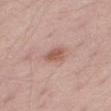The lesion was tiled from a total-body skin photograph and was not biopsied.
Automated tile analysis of the lesion measured a footprint of about 4 mm², an outline eccentricity of about 0.75 (0 = round, 1 = elongated), and two-axis asymmetry of about 0.3. And it measured a mean CIELAB color near L≈56 a*≈23 b*≈26, roughly 11 lightness units darker than nearby skin, and a lesion-to-skin contrast of about 7.5 (normalized; higher = more distinct). It also reported a border-irregularity rating of about 2.5/10 and a peripheral color-asymmetry measure near 0.5. The software also gave a lesion-detection confidence of about 100/100.
A 15 mm close-up extracted from a 3D total-body photography capture.
Approximately 2.5 mm at its widest.
From the right thigh.
The tile uses white-light illumination.
A male patient roughly 50 years of age.